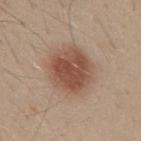  biopsy_status: not biopsied; imaged during a skin examination
  automated_metrics:
    border_irregularity_0_10: 1.5
    color_variation_0_10: 4.5
    peripheral_color_asymmetry: 1.5
    nevus_likeness_0_100: 100
    lesion_detection_confidence_0_100: 100
  lighting: white-light
  lesion_size:
    long_diameter_mm_approx: 5.5
  patient:
    sex: male
    age_approx: 30
  site: mid back
  image:
    source: total-body photography crop
    field_of_view_mm: 15